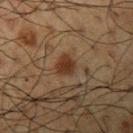Impression:
Recorded during total-body skin imaging; not selected for excision or biopsy.
Acquisition and patient details:
This is a cross-polarized tile. The lesion's longest dimension is about 2.5 mm. The lesion is located on the left upper arm. A 15 mm close-up tile from a total-body photography series done for melanoma screening. The subject is a male aged 58 to 62.